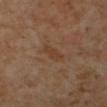Clinical summary:
A female patient, aged approximately 50. A 15 mm close-up tile from a total-body photography series done for melanoma screening. The tile uses cross-polarized illumination. The recorded lesion diameter is about 3.5 mm. From the leg.